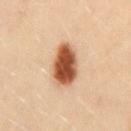Q: Was this lesion biopsied?
A: imaged on a skin check; not biopsied
Q: Lesion size?
A: ≈5 mm
Q: Who is the patient?
A: female, aged 28 to 32
Q: What did automated image analysis measure?
A: a footprint of about 12 mm², an eccentricity of roughly 0.8, and a symmetry-axis asymmetry near 0.15; a border-irregularity index near 1.5/10, internal color variation of about 5 on a 0–10 scale, and peripheral color asymmetry of about 1.5
Q: How was this image acquired?
A: ~15 mm crop, total-body skin-cancer survey
Q: Where on the body is the lesion?
A: the mid back
Q: How was the tile lit?
A: cross-polarized